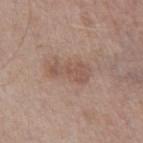workup: no biopsy performed (imaged during a skin exam)
anatomic site: the right thigh
illumination: white-light
patient: male, roughly 75 years of age
lesion diameter: ≈4.5 mm
image: ~15 mm tile from a whole-body skin photo